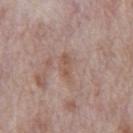This lesion was catalogued during total-body skin photography and was not selected for biopsy.
The tile uses white-light illumination.
The lesion-visualizer software estimated a nevus-likeness score of about 0/100 and a detector confidence of about 100 out of 100 that the crop contains a lesion.
Measured at roughly 3.5 mm in maximum diameter.
The lesion is located on the abdomen.
The patient is a male aged approximately 70.
A roughly 15 mm field-of-view crop from a total-body skin photograph.